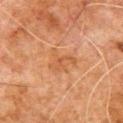Findings:
– follow-up — imaged on a skin check; not biopsied
– automated metrics — border irregularity of about 5.5 on a 0–10 scale, a color-variation rating of about 2.5/10, and a peripheral color-asymmetry measure near 1; a nevus-likeness score of about 0/100 and a detector confidence of about 100 out of 100 that the crop contains a lesion
– patient — male, in their 80s
– lesion size — about 3.5 mm
– image source — ~15 mm tile from a whole-body skin photo
– illumination — cross-polarized illumination
– location — the chest